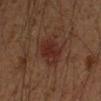Clinical impression:
Recorded during total-body skin imaging; not selected for excision or biopsy.
Clinical summary:
The recorded lesion diameter is about 4.5 mm. An algorithmic analysis of the crop reported a footprint of about 11 mm² and two-axis asymmetry of about 0.25. It also reported a mean CIELAB color near L≈25 a*≈19 b*≈21, roughly 6 lightness units darker than nearby skin, and a normalized lesion–skin contrast near 7. A male subject aged 48 to 52. Located on the left forearm. Captured under cross-polarized illumination. A close-up tile cropped from a whole-body skin photograph, about 15 mm across.